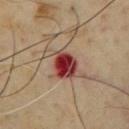<case>
<site>chest</site>
<lighting>cross-polarized</lighting>
<patient>
  <sex>male</sex>
  <age_approx>65</age_approx>
</patient>
<lesion_size>
  <long_diameter_mm_approx>4.5</long_diameter_mm_approx>
</lesion_size>
<image>
  <source>total-body photography crop</source>
  <field_of_view_mm>15</field_of_view_mm>
</image>
</case>